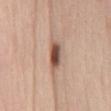follow-up — imaged on a skin check; not biopsied | acquisition — total-body-photography crop, ~15 mm field of view | tile lighting — white-light illumination | body site — the abdomen | patient — female, aged 48 to 52.